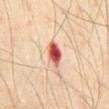Impression:
No biopsy was performed on this lesion — it was imaged during a full skin examination and was not determined to be concerning.
Clinical summary:
A 15 mm close-up tile from a total-body photography series done for melanoma screening. The subject is a male in their 70s. Located on the abdomen.